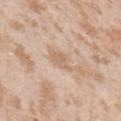This lesion was catalogued during total-body skin photography and was not selected for biopsy.
On the arm.
About 3 mm across.
A female patient, aged approximately 25.
A region of skin cropped from a whole-body photographic capture, roughly 15 mm wide.
Captured under white-light illumination.